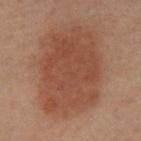Clinical impression: Imaged during a routine full-body skin examination; the lesion was not biopsied and no histopathology is available. Context: The lesion is located on the chest. Automated image analysis of the tile measured peripheral color asymmetry of about 1. And it measured a nevus-likeness score of about 100/100. A region of skin cropped from a whole-body photographic capture, roughly 15 mm wide. The subject is a male in their 60s.